On the chest; The lesion-visualizer software estimated an eccentricity of roughly 0.6 and a symmetry-axis asymmetry near 0.3. It also reported a classifier nevus-likeness of about 30/100; imaged with white-light lighting; a male patient approximately 80 years of age; a region of skin cropped from a whole-body photographic capture, roughly 15 mm wide: 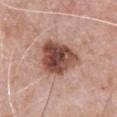Q: What is the histopathologic diagnosis?
A: an atypical intraepithelial melanocytic proliferation — an indeterminate (borderline) lesion